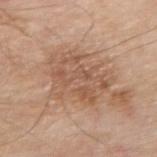biopsy_status: not biopsied; imaged during a skin examination